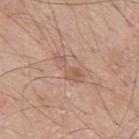A 15 mm crop from a total-body photograph taken for skin-cancer surveillance. Imaged with white-light lighting. On the left upper arm. Approximately 4 mm at its widest. The subject is a male roughly 60 years of age.